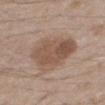The lesion was tiled from a total-body skin photograph and was not biopsied.
Longest diameter approximately 6.5 mm.
Cropped from a total-body skin-imaging series; the visible field is about 15 mm.
This is a white-light tile.
The lesion is located on the right thigh.
A male patient, in their mid- to late 60s.